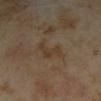No biopsy was performed on this lesion — it was imaged during a full skin examination and was not determined to be concerning.
A 15 mm close-up tile from a total-body photography series done for melanoma screening.
A female subject, roughly 35 years of age.
About 4 mm across.
This is a cross-polarized tile.
From the left forearm.